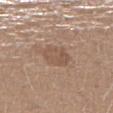Q: Was this lesion biopsied?
A: total-body-photography surveillance lesion; no biopsy
Q: What kind of image is this?
A: 15 mm crop, total-body photography
Q: Who is the patient?
A: female, about 35 years old
Q: Where on the body is the lesion?
A: the right lower leg